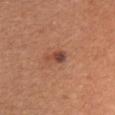Assessment: Imaged during a routine full-body skin examination; the lesion was not biopsied and no histopathology is available. Image and clinical context: The tile uses white-light illumination. The lesion's longest dimension is about 2.5 mm. The patient is a female aged around 35. A 15 mm crop from a total-body photograph taken for skin-cancer surveillance. From the front of the torso.